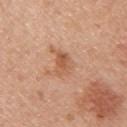Findings:
- workup · catalogued during a skin exam; not biopsied
- lighting · white-light illumination
- automated metrics · an area of roughly 5.5 mm², an outline eccentricity of about 0.7 (0 = round, 1 = elongated), and a symmetry-axis asymmetry near 0.35; an average lesion color of about L≈57 a*≈23 b*≈34 (CIELAB), roughly 9 lightness units darker than nearby skin, and a normalized lesion–skin contrast near 6.5; a border-irregularity rating of about 3.5/10, a color-variation rating of about 4/10, and peripheral color asymmetry of about 1.5
- diameter · ≈3 mm
- location · the right upper arm
- patient · female, in their 50s
- acquisition · 15 mm crop, total-body photography